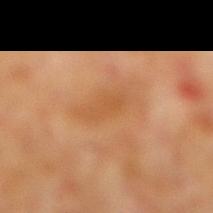Recorded during total-body skin imaging; not selected for excision or biopsy.
The lesion is located on the right lower leg.
A roughly 15 mm field-of-view crop from a total-body skin photograph.
Longest diameter approximately 3.5 mm.
Imaged with cross-polarized lighting.
The patient is a male aged 58 to 62.